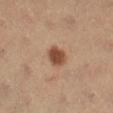Captured during whole-body skin photography for melanoma surveillance; the lesion was not biopsied. Measured at roughly 3 mm in maximum diameter. A 15 mm close-up extracted from a 3D total-body photography capture. The tile uses cross-polarized illumination. The lesion is on the right lower leg. A female patient, roughly 35 years of age.